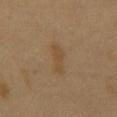No biopsy was performed on this lesion — it was imaged during a full skin examination and was not determined to be concerning. This is a cross-polarized tile. Located on the chest. A close-up tile cropped from a whole-body skin photograph, about 15 mm across. The patient is a male roughly 50 years of age.